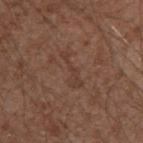Context:
The recorded lesion diameter is about 4 mm. The patient is a male in their mid- to late 70s. The lesion is located on the arm. A roughly 15 mm field-of-view crop from a total-body skin photograph.